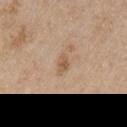About 2.5 mm across.
The tile uses white-light illumination.
Automated tile analysis of the lesion measured a footprint of about 3 mm² and a shape-asymmetry score of about 0.3 (0 = symmetric). And it measured a mean CIELAB color near L≈57 a*≈17 b*≈32 and roughly 9 lightness units darker than nearby skin. And it measured border irregularity of about 3 on a 0–10 scale and peripheral color asymmetry of about 0.5.
The patient is a male aged 73 to 77.
A close-up tile cropped from a whole-body skin photograph, about 15 mm across.
The lesion is on the right upper arm.